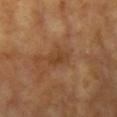Q: Lesion size?
A: ~3 mm (longest diameter)
Q: What is the anatomic site?
A: the right upper arm
Q: Patient demographics?
A: female, aged approximately 75
Q: What is the imaging modality?
A: total-body-photography crop, ~15 mm field of view
Q: Illumination type?
A: cross-polarized illumination